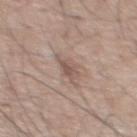Q: Was a biopsy performed?
A: catalogued during a skin exam; not biopsied
Q: How was the tile lit?
A: white-light illumination
Q: Where on the body is the lesion?
A: the back
Q: What is the imaging modality?
A: ~15 mm crop, total-body skin-cancer survey
Q: Patient demographics?
A: male, about 55 years old
Q: Lesion size?
A: about 3 mm
Q: Automated lesion metrics?
A: a lesion area of about 4.5 mm², an eccentricity of roughly 0.7, and a symmetry-axis asymmetry near 0.45; an average lesion color of about L≈54 a*≈16 b*≈23 (CIELAB) and roughly 9 lightness units darker than nearby skin; a within-lesion color-variation index near 2/10